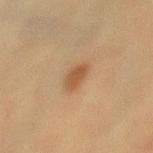Notes:
– automated metrics: a lesion area of about 5 mm² and an outline eccentricity of about 0.65 (0 = round, 1 = elongated); a color-variation rating of about 2/10; a nevus-likeness score of about 90/100 and a detector confidence of about 100 out of 100 that the crop contains a lesion
– imaging modality: ~15 mm crop, total-body skin-cancer survey
– location: the left lower leg
– subject: female, aged 38 to 42
– lighting: cross-polarized illumination
– diameter: about 2.5 mm